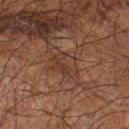{
  "biopsy_status": "not biopsied; imaged during a skin examination",
  "patient": {
    "sex": "male",
    "age_approx": 65
  },
  "site": "left forearm",
  "lighting": "cross-polarized",
  "image": {
    "source": "total-body photography crop",
    "field_of_view_mm": 15
  },
  "automated_metrics": {
    "area_mm2_approx": 7.0,
    "eccentricity": 0.65,
    "shape_asymmetry": 0.25,
    "vs_skin_darker_L": 5.0,
    "vs_skin_contrast_norm": 5.5,
    "border_irregularity_0_10": 3.0,
    "color_variation_0_10": 3.0,
    "peripheral_color_asymmetry": 1.0
  }
}